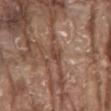Image and clinical context: About 2.5 mm across. A male patient aged around 80. From the chest. Automated tile analysis of the lesion measured an average lesion color of about L≈44 a*≈19 b*≈28 (CIELAB) and a normalized lesion–skin contrast near 6.5. It also reported internal color variation of about 1.5 on a 0–10 scale and a peripheral color-asymmetry measure near 0.5. It also reported a classifier nevus-likeness of about 0/100. Captured under white-light illumination. A roughly 15 mm field-of-view crop from a total-body skin photograph.